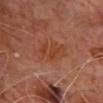subject: male, aged 63 to 67; lesion size: ~5.5 mm (longest diameter); location: the chest; imaging modality: ~15 mm tile from a whole-body skin photo; illumination: cross-polarized illumination.